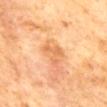| feature | finding |
|---|---|
| biopsy status | catalogued during a skin exam; not biopsied |
| TBP lesion metrics | a mean CIELAB color near L≈66 a*≈26 b*≈43 and a lesion–skin lightness drop of about 8; a border-irregularity index near 8/10 and a within-lesion color-variation index near 0/10; a nevus-likeness score of about 5/100 and lesion-presence confidence of about 100/100 |
| tile lighting | cross-polarized |
| size | ~3 mm (longest diameter) |
| patient | female, roughly 65 years of age |
| imaging modality | total-body-photography crop, ~15 mm field of view |
| site | the mid back |